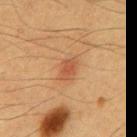Image and clinical context:
A lesion tile, about 15 mm wide, cut from a 3D total-body photograph. Imaged with cross-polarized lighting. From the abdomen. The patient is a male in their 60s.